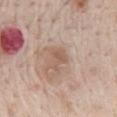  biopsy_status: not biopsied; imaged during a skin examination
  automated_metrics:
    area_mm2_approx: 5.0
    eccentricity: 0.7
    shape_asymmetry: 0.4
    cielab_L: 57
    cielab_a: 18
    cielab_b: 27
    vs_skin_darker_L: 8.0
    vs_skin_contrast_norm: 5.5
  image:
    source: total-body photography crop
    field_of_view_mm: 15
  patient:
    sex: male
    age_approx: 65
  site: mid back